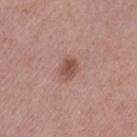{"site": "left thigh", "lesion_size": {"long_diameter_mm_approx": 2.5}, "patient": {"sex": "female", "age_approx": 60}, "image": {"source": "total-body photography crop", "field_of_view_mm": 15}}